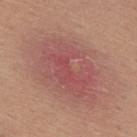Impression:
No biopsy was performed on this lesion — it was imaged during a full skin examination and was not determined to be concerning.